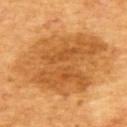Assessment:
Captured during whole-body skin photography for melanoma surveillance; the lesion was not biopsied.
Acquisition and patient details:
Imaged with cross-polarized lighting. On the upper back. This image is a 15 mm lesion crop taken from a total-body photograph. An algorithmic analysis of the crop reported an automated nevus-likeness rating near 70 out of 100 and a lesion-detection confidence of about 100/100. The lesion's longest dimension is about 11.5 mm. A male subject, roughly 65 years of age.